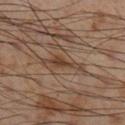<tbp_lesion>
  <biopsy_status>not biopsied; imaged during a skin examination</biopsy_status>
  <site>right lower leg</site>
  <image>
    <source>total-body photography crop</source>
    <field_of_view_mm>15</field_of_view_mm>
  </image>
  <lesion_size>
    <long_diameter_mm_approx>3.5</long_diameter_mm_approx>
  </lesion_size>
  <lighting>cross-polarized</lighting>
  <patient>
    <sex>male</sex>
    <age_approx>55</age_approx>
  </patient>
</tbp_lesion>